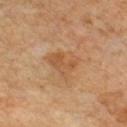Case summary:
– biopsy status · imaged on a skin check; not biopsied
– diameter · ~4 mm (longest diameter)
– tile lighting · cross-polarized
– automated metrics · a footprint of about 9.5 mm² and a symmetry-axis asymmetry near 0.4; a border-irregularity rating of about 4/10
– image source · total-body-photography crop, ~15 mm field of view
– patient · male, aged around 60
– anatomic site · the chest